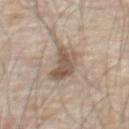  biopsy_status: not biopsied; imaged during a skin examination
  patient:
    sex: male
    age_approx: 80
  image:
    source: total-body photography crop
    field_of_view_mm: 15
  site: chest